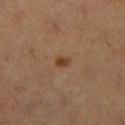Impression: This lesion was catalogued during total-body skin photography and was not selected for biopsy. Acquisition and patient details: A female patient approximately 30 years of age. The lesion is located on the right lower leg. This image is a 15 mm lesion crop taken from a total-body photograph.